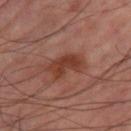| key | value |
|---|---|
| notes | no biopsy performed (imaged during a skin exam) |
| lighting | cross-polarized |
| location | the right thigh |
| imaging modality | total-body-photography crop, ~15 mm field of view |